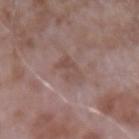Notes:
– notes: no biopsy performed (imaged during a skin exam)
– image source: ~15 mm crop, total-body skin-cancer survey
– patient: male, aged around 65
– site: the left forearm
– tile lighting: white-light illumination
– automated lesion analysis: a border-irregularity index near 4/10, internal color variation of about 3.5 on a 0–10 scale, and peripheral color asymmetry of about 1.5; lesion-presence confidence of about 100/100
– lesion diameter: ≈4 mm